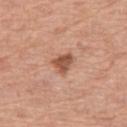{
  "biopsy_status": "not biopsied; imaged during a skin examination",
  "site": "leg",
  "patient": {
    "sex": "female",
    "age_approx": 60
  },
  "lesion_size": {
    "long_diameter_mm_approx": 2.5
  },
  "automated_metrics": {
    "cielab_L": 53,
    "cielab_a": 24,
    "cielab_b": 31,
    "vs_skin_darker_L": 13.0,
    "vs_skin_contrast_norm": 9.0
  },
  "image": {
    "source": "total-body photography crop",
    "field_of_view_mm": 15
  }
}